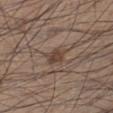Q: Was a biopsy performed?
A: catalogued during a skin exam; not biopsied
Q: What kind of image is this?
A: ~15 mm crop, total-body skin-cancer survey
Q: Lesion location?
A: the left lower leg
Q: How was the tile lit?
A: white-light illumination
Q: Patient demographics?
A: male, roughly 35 years of age
Q: How large is the lesion?
A: ≈3 mm
Q: Automated lesion metrics?
A: a shape eccentricity near 0.7 and a symmetry-axis asymmetry near 0.4